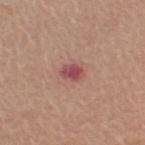Q: Was a biopsy performed?
A: imaged on a skin check; not biopsied
Q: How was the tile lit?
A: white-light illumination
Q: Patient demographics?
A: male, in their 80s
Q: What is the anatomic site?
A: the right upper arm
Q: How was this image acquired?
A: total-body-photography crop, ~15 mm field of view